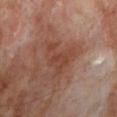<case>
<biopsy_status>not biopsied; imaged during a skin examination</biopsy_status>
<image>
  <source>total-body photography crop</source>
  <field_of_view_mm>15</field_of_view_mm>
</image>
<lesion_size>
  <long_diameter_mm_approx>5.0</long_diameter_mm_approx>
</lesion_size>
<site>left lower leg</site>
<patient>
  <sex>male</sex>
  <age_approx>70</age_approx>
</patient>
<lighting>cross-polarized</lighting>
<automated_metrics>
  <border_irregularity_0_10>6.0</border_irregularity_0_10>
  <color_variation_0_10>2.5</color_variation_0_10>
</automated_metrics>
</case>